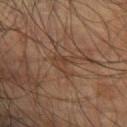{"biopsy_status": "not biopsied; imaged during a skin examination", "site": "right upper arm", "patient": {"sex": "male", "age_approx": 75}, "image": {"source": "total-body photography crop", "field_of_view_mm": 15}, "lighting": "cross-polarized"}